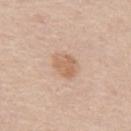- biopsy status — no biopsy performed (imaged during a skin exam)
- illumination — white-light
- imaging modality — 15 mm crop, total-body photography
- location — the back
- subject — male, aged around 60
- TBP lesion metrics — an area of roughly 6.5 mm² and a shape-asymmetry score of about 0.2 (0 = symmetric)